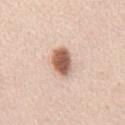workup: no biopsy performed (imaged during a skin exam)
tile lighting: white-light illumination
body site: the chest
subject: male, in their mid- to late 40s
image source: ~15 mm crop, total-body skin-cancer survey
image-analysis metrics: an area of roughly 8 mm², an outline eccentricity of about 0.8 (0 = round, 1 = elongated), and a shape-asymmetry score of about 0.15 (0 = symmetric); a classifier nevus-likeness of about 100/100 and lesion-presence confidence of about 100/100
diameter: about 4 mm